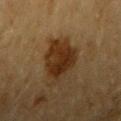Q: Was a biopsy performed?
A: imaged on a skin check; not biopsied
Q: What lighting was used for the tile?
A: cross-polarized illumination
Q: What is the anatomic site?
A: the right upper arm
Q: What is the imaging modality?
A: ~15 mm crop, total-body skin-cancer survey
Q: What is the lesion's diameter?
A: ~6 mm (longest diameter)
Q: Who is the patient?
A: male, in their mid- to late 80s or older